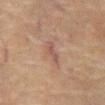Q: How was this image acquired?
A: ~15 mm crop, total-body skin-cancer survey
Q: Lesion size?
A: about 3 mm
Q: What are the patient's age and sex?
A: male, aged around 75
Q: Where on the body is the lesion?
A: the front of the torso